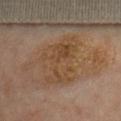Impression:
Recorded during total-body skin imaging; not selected for excision or biopsy.
Context:
Cropped from a whole-body photographic skin survey; the tile spans about 15 mm. The lesion is on the chest. A female patient aged around 60. An algorithmic analysis of the crop reported a classifier nevus-likeness of about 0/100 and a detector confidence of about 90 out of 100 that the crop contains a lesion. Approximately 6 mm at its widest. This is a cross-polarized tile.